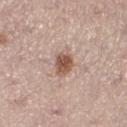The lesion was photographed on a routine skin check and not biopsied; there is no pathology result.
This is a white-light tile.
The patient is a female roughly 65 years of age.
A 15 mm close-up tile from a total-body photography series done for melanoma screening.
On the right lower leg.